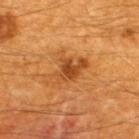Q: Was a biopsy performed?
A: total-body-photography surveillance lesion; no biopsy